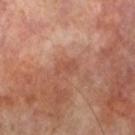Findings:
– follow-up · imaged on a skin check; not biopsied
– imaging modality · total-body-photography crop, ~15 mm field of view
– lesion size · ≈2.5 mm
– tile lighting · cross-polarized
– subject · male, about 70 years old
– body site · the left lower leg